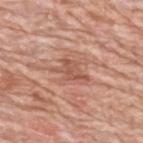{
  "biopsy_status": "not biopsied; imaged during a skin examination",
  "site": "upper back",
  "automated_metrics": {
    "area_mm2_approx": 5.5,
    "eccentricity": 0.8,
    "border_irregularity_0_10": 5.5,
    "color_variation_0_10": 2.5,
    "peripheral_color_asymmetry": 1.0
  },
  "image": {
    "source": "total-body photography crop",
    "field_of_view_mm": 15
  },
  "patient": {
    "sex": "male",
    "age_approx": 80
  }
}